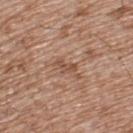Q: Was this lesion biopsied?
A: imaged on a skin check; not biopsied
Q: Patient demographics?
A: male, aged around 55
Q: How was this image acquired?
A: ~15 mm tile from a whole-body skin photo
Q: Lesion location?
A: the back